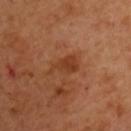Image and clinical context:
A roughly 15 mm field-of-view crop from a total-body skin photograph. A male subject, aged 48–52. From the chest.